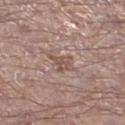Captured during whole-body skin photography for melanoma surveillance; the lesion was not biopsied.
A male subject, in their mid-60s.
On the left lower leg.
A 15 mm close-up extracted from a 3D total-body photography capture.